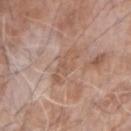<tbp_lesion>
<biopsy_status>not biopsied; imaged during a skin examination</biopsy_status>
<site>right forearm</site>
<patient>
  <sex>male</sex>
  <age_approx>75</age_approx>
</patient>
<lesion_size>
  <long_diameter_mm_approx>4.0</long_diameter_mm_approx>
</lesion_size>
<image>
  <source>total-body photography crop</source>
  <field_of_view_mm>15</field_of_view_mm>
</image>
</tbp_lesion>